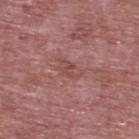Clinical impression: No biopsy was performed on this lesion — it was imaged during a full skin examination and was not determined to be concerning. Acquisition and patient details: Longest diameter approximately 3.5 mm. Automated tile analysis of the lesion measured an eccentricity of roughly 0.85. The software also gave a lesion color around L≈48 a*≈24 b*≈24 in CIELAB and a normalized border contrast of about 5.5. The analysis additionally found a border-irregularity index near 3.5/10, internal color variation of about 3 on a 0–10 scale, and peripheral color asymmetry of about 1. A 15 mm crop from a total-body photograph taken for skin-cancer surveillance. A male patient, aged around 75. The lesion is on the upper back.